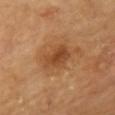* workup — catalogued during a skin exam; not biopsied
* lesion size — ~4.5 mm (longest diameter)
* patient — female, aged 63 to 67
* anatomic site — the chest
* image source — ~15 mm crop, total-body skin-cancer survey